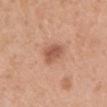Imaged during a routine full-body skin examination; the lesion was not biopsied and no histopathology is available.
Cropped from a total-body skin-imaging series; the visible field is about 15 mm.
A female subject aged around 40.
The tile uses white-light illumination.
About 3 mm across.
The lesion is located on the chest.